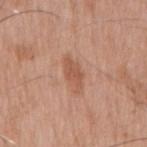| key | value |
|---|---|
| workup | total-body-photography surveillance lesion; no biopsy |
| lesion diameter | ≈4 mm |
| lighting | white-light illumination |
| patient | male, about 75 years old |
| anatomic site | the mid back |
| image source | ~15 mm crop, total-body skin-cancer survey |
| automated metrics | a border-irregularity index near 3.5/10, internal color variation of about 1.5 on a 0–10 scale, and a peripheral color-asymmetry measure near 0.5; an automated nevus-likeness rating near 15 out of 100 and a lesion-detection confidence of about 100/100 |